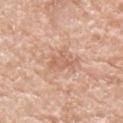The lesion was photographed on a routine skin check and not biopsied; there is no pathology result. The patient is a male aged 78 to 82. The lesion is located on the right upper arm. Measured at roughly 3.5 mm in maximum diameter. A roughly 15 mm field-of-view crop from a total-body skin photograph.